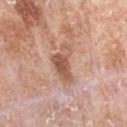No biopsy was performed on this lesion — it was imaged during a full skin examination and was not determined to be concerning. Automated image analysis of the tile measured a classifier nevus-likeness of about 0/100 and a lesion-detection confidence of about 100/100. Cropped from a whole-body photographic skin survey; the tile spans about 15 mm. Longest diameter approximately 5 mm. A female patient in their mid-70s. The lesion is located on the left forearm.